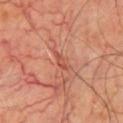Captured during whole-body skin photography for melanoma surveillance; the lesion was not biopsied.
The total-body-photography lesion software estimated an area of roughly 2.5 mm², an eccentricity of roughly 0.95, and two-axis asymmetry of about 0.4. And it measured a classifier nevus-likeness of about 0/100 and lesion-presence confidence of about 50/100.
A male subject, in their 70s.
A 15 mm crop from a total-body photograph taken for skin-cancer surveillance.
About 3 mm across.
Located on the chest.